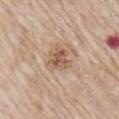Assessment:
Recorded during total-body skin imaging; not selected for excision or biopsy.
Context:
Imaged with white-light lighting. A male patient, aged approximately 65. The recorded lesion diameter is about 3.5 mm. Located on the right upper arm. This image is a 15 mm lesion crop taken from a total-body photograph.